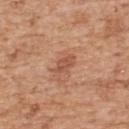Assessment:
This lesion was catalogued during total-body skin photography and was not selected for biopsy.
Clinical summary:
Located on the upper back. A close-up tile cropped from a whole-body skin photograph, about 15 mm across. Longest diameter approximately 2.5 mm. The tile uses white-light illumination. A male patient aged 58–62.